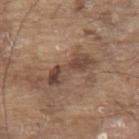This lesion was catalogued during total-body skin photography and was not selected for biopsy. The lesion-visualizer software estimated a lesion area of about 9.5 mm², a shape eccentricity near 0.95, and a shape-asymmetry score of about 0.35 (0 = symmetric). It also reported border irregularity of about 5 on a 0–10 scale and a color-variation rating of about 6.5/10. A male patient approximately 80 years of age. Cropped from a total-body skin-imaging series; the visible field is about 15 mm. Longest diameter approximately 6 mm. This is a white-light tile. On the upper back.